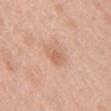{"biopsy_status": "not biopsied; imaged during a skin examination", "automated_metrics": {"area_mm2_approx": 4.5, "eccentricity": 0.75, "shape_asymmetry": 0.25, "cielab_L": 63, "cielab_a": 22, "cielab_b": 32, "vs_skin_darker_L": 8.0, "vs_skin_contrast_norm": 5.5}, "lighting": "white-light", "image": {"source": "total-body photography crop", "field_of_view_mm": 15}, "patient": {"sex": "male", "age_approx": 60}, "site": "right upper arm", "lesion_size": {"long_diameter_mm_approx": 3.0}}